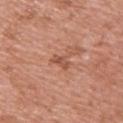| feature | finding |
|---|---|
| follow-up | total-body-photography surveillance lesion; no biopsy |
| lesion diameter | about 2.5 mm |
| tile lighting | white-light illumination |
| location | the upper back |
| imaging modality | ~15 mm tile from a whole-body skin photo |
| patient | female, about 40 years old |